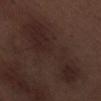Assessment:
Recorded during total-body skin imaging; not selected for excision or biopsy.
Background:
The lesion is located on the leg. A close-up tile cropped from a whole-body skin photograph, about 15 mm across. A male patient about 70 years old.